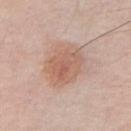No biopsy was performed on this lesion — it was imaged during a full skin examination and was not determined to be concerning. Imaged with white-light lighting. Cropped from a total-body skin-imaging series; the visible field is about 15 mm. About 5 mm across. The lesion is on the chest. A male patient, aged around 55.